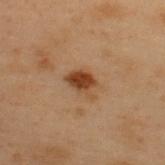Recorded during total-body skin imaging; not selected for excision or biopsy.
The lesion's longest dimension is about 3.5 mm.
The tile uses cross-polarized illumination.
This image is a 15 mm lesion crop taken from a total-body photograph.
A male patient, aged approximately 55.
The lesion is on the upper back.